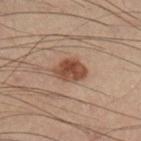notes: imaged on a skin check; not biopsied
subject: male, in their mid- to late 50s
body site: the right lower leg
acquisition: ~15 mm tile from a whole-body skin photo
diameter: ≈3.5 mm